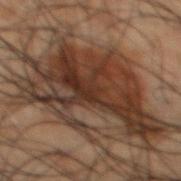On the mid back.
The recorded lesion diameter is about 12.5 mm.
Imaged with cross-polarized lighting.
The subject is a male roughly 50 years of age.
A close-up tile cropped from a whole-body skin photograph, about 15 mm across.